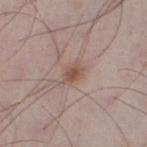This lesion was catalogued during total-body skin photography and was not selected for biopsy. The lesion is on the left lower leg. The patient is a male aged 48–52. A 15 mm close-up tile from a total-body photography series done for melanoma screening. Measured at roughly 3 mm in maximum diameter. This is a white-light tile.